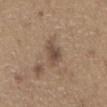* patient — male, aged around 65
* image source — ~15 mm crop, total-body skin-cancer survey
* body site — the abdomen